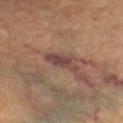Recorded during total-body skin imaging; not selected for excision or biopsy.
A 15 mm close-up extracted from a 3D total-body photography capture.
Measured at roughly 4.5 mm in maximum diameter.
A female patient aged around 70.
Captured under cross-polarized illumination.
Located on the right lower leg.